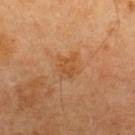Captured during whole-body skin photography for melanoma surveillance; the lesion was not biopsied. This image is a 15 mm lesion crop taken from a total-body photograph. The lesion is on the upper back. The lesion's longest dimension is about 3 mm. The tile uses cross-polarized illumination. The subject is a male about 60 years old.